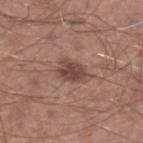Q: Lesion location?
A: the right lower leg
Q: How large is the lesion?
A: ~3.5 mm (longest diameter)
Q: How was the tile lit?
A: white-light illumination
Q: What are the patient's age and sex?
A: male, in their mid-60s
Q: What is the imaging modality?
A: total-body-photography crop, ~15 mm field of view